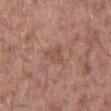biopsy status: imaged on a skin check; not biopsied
imaging modality: total-body-photography crop, ~15 mm field of view
diameter: ≈3 mm
anatomic site: the mid back
tile lighting: white-light illumination
automated metrics: border irregularity of about 4.5 on a 0–10 scale and a color-variation rating of about 2/10; a nevus-likeness score of about 0/100 and a lesion-detection confidence of about 100/100
subject: male, aged approximately 55